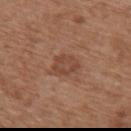Q: What are the patient's age and sex?
A: female, aged 73 to 77
Q: What did automated image analysis measure?
A: a lesion color around L≈45 a*≈21 b*≈29 in CIELAB, a lesion–skin lightness drop of about 8, and a normalized lesion–skin contrast near 6; a border-irregularity index near 3/10, a color-variation rating of about 2.5/10, and radial color variation of about 1
Q: What is the imaging modality?
A: ~15 mm crop, total-body skin-cancer survey
Q: Lesion location?
A: the upper back
Q: What lighting was used for the tile?
A: white-light